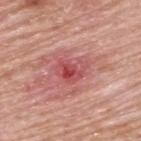Assessment: Captured during whole-body skin photography for melanoma surveillance; the lesion was not biopsied. Acquisition and patient details: A male patient in their 80s. On the upper back. Imaged with white-light lighting. This image is a 15 mm lesion crop taken from a total-body photograph.